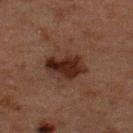Q: Was a biopsy performed?
A: no biopsy performed (imaged during a skin exam)
Q: How was this image acquired?
A: ~15 mm tile from a whole-body skin photo
Q: What is the lesion's diameter?
A: about 4.5 mm
Q: What is the anatomic site?
A: the upper back
Q: What did automated image analysis measure?
A: a lesion area of about 10 mm², an outline eccentricity of about 0.75 (0 = round, 1 = elongated), and two-axis asymmetry of about 0.3; about 10 CIELAB-L* units darker than the surrounding skin and a normalized lesion–skin contrast near 11.5; a classifier nevus-likeness of about 70/100 and lesion-presence confidence of about 100/100
Q: Patient demographics?
A: male, in their 50s